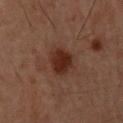{
  "biopsy_status": "not biopsied; imaged during a skin examination",
  "patient": {
    "sex": "male",
    "age_approx": 60
  },
  "lighting": "cross-polarized",
  "automated_metrics": {
    "eccentricity": 0.8,
    "shape_asymmetry": 0.2,
    "nevus_likeness_0_100": 85,
    "lesion_detection_confidence_0_100": 100
  },
  "site": "mid back",
  "lesion_size": {
    "long_diameter_mm_approx": 4.0
  },
  "image": {
    "source": "total-body photography crop",
    "field_of_view_mm": 15
  }
}